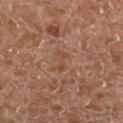Impression:
This lesion was catalogued during total-body skin photography and was not selected for biopsy.
Image and clinical context:
Longest diameter approximately 3 mm. The tile uses cross-polarized illumination. The subject is a male aged 53–57. On the left lower leg. A 15 mm close-up extracted from a 3D total-body photography capture. Automated tile analysis of the lesion measured a footprint of about 2.5 mm² and two-axis asymmetry of about 0.6. The analysis additionally found an average lesion color of about L≈45 a*≈22 b*≈30 (CIELAB), a lesion–skin lightness drop of about 5, and a lesion-to-skin contrast of about 5 (normalized; higher = more distinct). The software also gave a border-irregularity index near 7/10, internal color variation of about 0 on a 0–10 scale, and peripheral color asymmetry of about 0. The software also gave a classifier nevus-likeness of about 0/100 and a detector confidence of about 85 out of 100 that the crop contains a lesion.